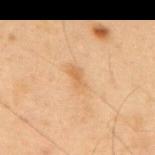Clinical impression:
The lesion was tiled from a total-body skin photograph and was not biopsied.
Clinical summary:
Imaged with cross-polarized lighting. The subject is a male aged approximately 55. The lesion is on the mid back. A 15 mm close-up tile from a total-body photography series done for melanoma screening.